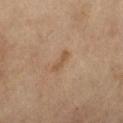Recorded during total-body skin imaging; not selected for excision or biopsy. The patient is a female in their 60s. The tile uses cross-polarized illumination. The recorded lesion diameter is about 3 mm. On the left thigh. A 15 mm crop from a total-body photograph taken for skin-cancer surveillance. An algorithmic analysis of the crop reported a shape eccentricity near 0.95 and a shape-asymmetry score of about 0.45 (0 = symmetric). The analysis additionally found a nevus-likeness score of about 0/100 and a lesion-detection confidence of about 100/100.